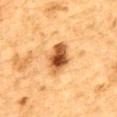Part of a total-body skin-imaging series; this lesion was reviewed on a skin check and was not flagged for biopsy. From the mid back. A roughly 15 mm field-of-view crop from a total-body skin photograph. Measured at roughly 5 mm in maximum diameter. The tile uses cross-polarized illumination. Automated image analysis of the tile measured an area of roughly 9 mm² and an eccentricity of roughly 0.85. The software also gave about 17 CIELAB-L* units darker than the surrounding skin and a normalized lesion–skin contrast near 11.5. It also reported a nevus-likeness score of about 95/100 and a detector confidence of about 100 out of 100 that the crop contains a lesion. The patient is a male in their mid-80s or older.